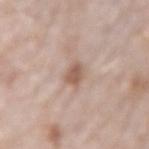No biopsy was performed on this lesion — it was imaged during a full skin examination and was not determined to be concerning.
About 3 mm across.
The lesion is on the left forearm.
The patient is a male aged 73–77.
A 15 mm crop from a total-body photograph taken for skin-cancer surveillance.
The tile uses white-light illumination.